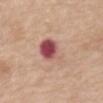Q: Is there a histopathology result?
A: catalogued during a skin exam; not biopsied
Q: Patient demographics?
A: female, about 65 years old
Q: How was this image acquired?
A: ~15 mm tile from a whole-body skin photo
Q: What lighting was used for the tile?
A: white-light illumination
Q: Automated lesion metrics?
A: an area of roughly 10 mm², an eccentricity of roughly 0.6, and a symmetry-axis asymmetry near 0.2; an average lesion color of about L≈53 a*≈26 b*≈23 (CIELAB); a classifier nevus-likeness of about 0/100
Q: Lesion location?
A: the abdomen